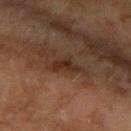workup = total-body-photography surveillance lesion; no biopsy
patient = female, approximately 60 years of age
illumination = cross-polarized
imaging modality = ~15 mm tile from a whole-body skin photo
diameter = ~3 mm (longest diameter)
anatomic site = the left forearm